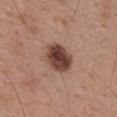<tbp_lesion>
<biopsy_status>not biopsied; imaged during a skin examination</biopsy_status>
<lighting>white-light</lighting>
<automated_metrics>
  <eccentricity>0.55</eccentricity>
  <border_irregularity_0_10>1.5</border_irregularity_0_10>
  <color_variation_0_10>5.5</color_variation_0_10>
  <peripheral_color_asymmetry>2.0</peripheral_color_asymmetry>
</automated_metrics>
<patient>
  <sex>male</sex>
  <age_approx>55</age_approx>
</patient>
<image>
  <source>total-body photography crop</source>
  <field_of_view_mm>15</field_of_view_mm>
</image>
<site>mid back</site>
<lesion_size>
  <long_diameter_mm_approx>4.0</long_diameter_mm_approx>
</lesion_size>
</tbp_lesion>